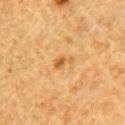  biopsy_status: not biopsied; imaged during a skin examination
  site: right upper arm
  image:
    source: total-body photography crop
    field_of_view_mm: 15
  automated_metrics:
    eccentricity: 0.85
    shape_asymmetry: 0.55
    color_variation_0_10: 1.0
    nevus_likeness_0_100: 30
  patient:
    sex: male
    age_approx: 85+
  lesion_size:
    long_diameter_mm_approx: 2.5